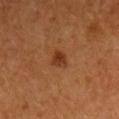automated metrics: a lesion-to-skin contrast of about 7.5 (normalized; higher = more distinct); a within-lesion color-variation index near 2.5/10 and peripheral color asymmetry of about 1; an automated nevus-likeness rating near 95 out of 100 and a lesion-detection confidence of about 100/100 | patient: male, in their 60s | location: the right upper arm | size: about 2.5 mm | acquisition: ~15 mm crop, total-body skin-cancer survey.